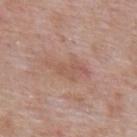{
  "biopsy_status": "not biopsied; imaged during a skin examination",
  "patient": {
    "sex": "male",
    "age_approx": 55
  },
  "site": "upper back",
  "lesion_size": {
    "long_diameter_mm_approx": 5.5
  },
  "image": {
    "source": "total-body photography crop",
    "field_of_view_mm": 15
  }
}